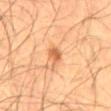From the abdomen. A 15 mm crop from a total-body photograph taken for skin-cancer surveillance. A male patient roughly 60 years of age.